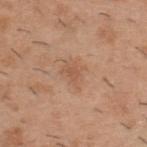Part of a total-body skin-imaging series; this lesion was reviewed on a skin check and was not flagged for biopsy.
A male patient, in their 40s.
Located on the back.
Cropped from a total-body skin-imaging series; the visible field is about 15 mm.